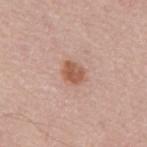{
  "automated_metrics": {
    "area_mm2_approx": 5.0,
    "eccentricity": 0.7,
    "shape_asymmetry": 0.2,
    "border_irregularity_0_10": 2.0,
    "color_variation_0_10": 3.0,
    "peripheral_color_asymmetry": 1.0,
    "lesion_detection_confidence_0_100": 100
  },
  "patient": {
    "sex": "male",
    "age_approx": 60
  },
  "image": {
    "source": "total-body photography crop",
    "field_of_view_mm": 15
  },
  "lesion_size": {
    "long_diameter_mm_approx": 3.0
  },
  "site": "mid back"
}